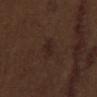  biopsy_status: not biopsied; imaged during a skin examination
  image:
    source: total-body photography crop
    field_of_view_mm: 15
  lesion_size:
    long_diameter_mm_approx: 2.5
  lighting: white-light
  patient:
    sex: male
    age_approx: 70
  site: mid back